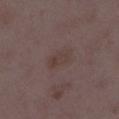Clinical impression:
Recorded during total-body skin imaging; not selected for excision or biopsy.
Context:
A female subject in their mid- to late 30s. The lesion is on the right thigh. About 3 mm across. This image is a 15 mm lesion crop taken from a total-body photograph.